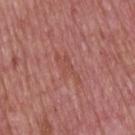Notes:
• follow-up · catalogued during a skin exam; not biopsied
• TBP lesion metrics · a footprint of about 4 mm², an outline eccentricity of about 0.9 (0 = round, 1 = elongated), and two-axis asymmetry of about 0.5; border irregularity of about 8 on a 0–10 scale, internal color variation of about 0.5 on a 0–10 scale, and a peripheral color-asymmetry measure near 0
• imaging modality · total-body-photography crop, ~15 mm field of view
• site · the upper back
• patient · male, aged around 75